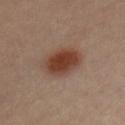Context:
The patient is a male about 35 years old. This is a cross-polarized tile. Cropped from a whole-body photographic skin survey; the tile spans about 15 mm. Approximately 4.5 mm at its widest. Located on the chest.